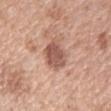notes — catalogued during a skin exam; not biopsied | automated metrics — a lesion area of about 9 mm² and an outline eccentricity of about 0.35 (0 = round, 1 = elongated); a lesion color around L≈55 a*≈22 b*≈27 in CIELAB, roughly 13 lightness units darker than nearby skin, and a normalized lesion–skin contrast near 8.5; a border-irregularity index near 1.5/10, a color-variation rating of about 3/10, and a peripheral color-asymmetry measure near 1 | site — the right forearm | size — ≈3.5 mm | lighting — white-light | image source — total-body-photography crop, ~15 mm field of view | patient — female, approximately 60 years of age.